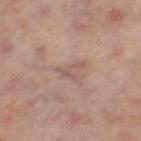follow-up: no biopsy performed (imaged during a skin exam) | site: the left thigh | tile lighting: cross-polarized | imaging modality: ~15 mm tile from a whole-body skin photo | automated lesion analysis: a footprint of about 3 mm², an eccentricity of roughly 0.85, and a shape-asymmetry score of about 0.75 (0 = symmetric); a border-irregularity rating of about 8.5/10, internal color variation of about 0 on a 0–10 scale, and radial color variation of about 0; an automated nevus-likeness rating near 0 out of 100 and a lesion-detection confidence of about 70/100 | patient: female, approximately 50 years of age.